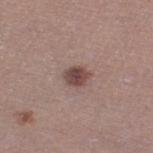Imaged during a routine full-body skin examination; the lesion was not biopsied and no histopathology is available. Measured at roughly 2.5 mm in maximum diameter. A female subject, roughly 35 years of age. Located on the left leg. This image is a 15 mm lesion crop taken from a total-body photograph. This is a white-light tile.